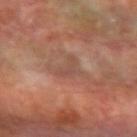Findings:
- follow-up — imaged on a skin check; not biopsied
- body site — the left forearm
- subject — male, aged approximately 70
- image — 15 mm crop, total-body photography